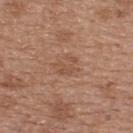Part of a total-body skin-imaging series; this lesion was reviewed on a skin check and was not flagged for biopsy. On the upper back. A female subject aged 38 to 42. Imaged with white-light lighting. Cropped from a total-body skin-imaging series; the visible field is about 15 mm.